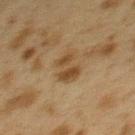| key | value |
|---|---|
| workup | imaged on a skin check; not biopsied |
| illumination | cross-polarized |
| body site | the upper back |
| patient | female, in their 40s |
| diameter | ~3.5 mm (longest diameter) |
| TBP lesion metrics | an area of roughly 7.5 mm² and two-axis asymmetry of about 0.35; an average lesion color of about L≈38 a*≈13 b*≈30 (CIELAB), a lesion–skin lightness drop of about 8, and a normalized lesion–skin contrast near 7.5; a border-irregularity index near 4.5/10, internal color variation of about 3.5 on a 0–10 scale, and peripheral color asymmetry of about 1; a classifier nevus-likeness of about 5/100 and lesion-presence confidence of about 100/100 |
| imaging modality | ~15 mm tile from a whole-body skin photo |